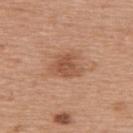* tile lighting: white-light illumination
* TBP lesion metrics: a lesion area of about 7.5 mm², an outline eccentricity of about 0.5 (0 = round, 1 = elongated), and two-axis asymmetry of about 0.35
* subject: female, aged approximately 60
* site: the upper back
* lesion diameter: about 3.5 mm
* image: ~15 mm crop, total-body skin-cancer survey